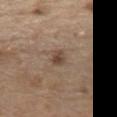notes: imaged on a skin check; not biopsied | site: the chest | subject: male, aged around 70 | imaging modality: total-body-photography crop, ~15 mm field of view.